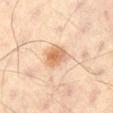{"biopsy_status": "not biopsied; imaged during a skin examination", "lesion_size": {"long_diameter_mm_approx": 3.5}, "lighting": "cross-polarized", "image": {"source": "total-body photography crop", "field_of_view_mm": 15}, "patient": {"sex": "male", "age_approx": 60}, "automated_metrics": {"area_mm2_approx": 7.0, "eccentricity": 0.65, "shape_asymmetry": 0.2}, "site": "right thigh"}